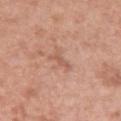The lesion was photographed on a routine skin check and not biopsied; there is no pathology result.
A female subject, in their 60s.
Located on the upper back.
Cropped from a total-body skin-imaging series; the visible field is about 15 mm.
Imaged with white-light lighting.
Approximately 3 mm at its widest.